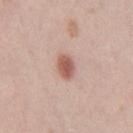Context: An algorithmic analysis of the crop reported border irregularity of about 2 on a 0–10 scale, a color-variation rating of about 3.5/10, and peripheral color asymmetry of about 1. The lesion is located on the right thigh. Approximately 3 mm at its widest. The tile uses white-light illumination. A female subject aged approximately 25. Cropped from a whole-body photographic skin survey; the tile spans about 15 mm. Conclusion: Histopathologically confirmed as a dysplastic (Clark) nevus.